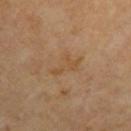Q: Was a biopsy performed?
A: total-body-photography surveillance lesion; no biopsy
Q: Where on the body is the lesion?
A: the left upper arm
Q: How was this image acquired?
A: 15 mm crop, total-body photography
Q: How was the tile lit?
A: cross-polarized
Q: Patient demographics?
A: in their mid- to late 60s